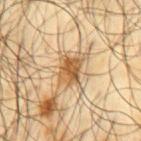Q: What did automated image analysis measure?
A: an average lesion color of about L≈55 a*≈18 b*≈38 (CIELAB), a lesion–skin lightness drop of about 13, and a normalized border contrast of about 9.5; a border-irregularity rating of about 2.5/10, a within-lesion color-variation index near 5/10, and a peripheral color-asymmetry measure near 2; an automated nevus-likeness rating near 90 out of 100 and lesion-presence confidence of about 100/100
Q: What is the anatomic site?
A: the mid back
Q: Illumination type?
A: cross-polarized
Q: How was this image acquired?
A: 15 mm crop, total-body photography
Q: Patient demographics?
A: male, aged 63 to 67